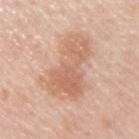Q: Is there a histopathology result?
A: total-body-photography surveillance lesion; no biopsy
Q: What lighting was used for the tile?
A: white-light illumination
Q: Automated lesion metrics?
A: a lesion area of about 27 mm² and two-axis asymmetry of about 0.4; border irregularity of about 5.5 on a 0–10 scale and a peripheral color-asymmetry measure near 1.5
Q: Where on the body is the lesion?
A: the right upper arm
Q: What kind of image is this?
A: 15 mm crop, total-body photography
Q: Patient demographics?
A: male, approximately 60 years of age